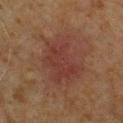<lesion>
<image>
  <source>total-body photography crop</source>
  <field_of_view_mm>15</field_of_view_mm>
</image>
<site>chest</site>
<patient>
  <sex>male</sex>
  <age_approx>60</age_approx>
</patient>
</lesion>